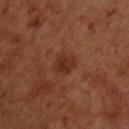Cropped from a whole-body photographic skin survey; the tile spans about 15 mm.
The lesion is located on the chest.
This is a cross-polarized tile.
A male subject aged 48–52.